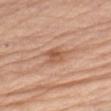anatomic site = the left upper arm
automated lesion analysis = a footprint of about 5 mm², a shape eccentricity near 0.8, and a symmetry-axis asymmetry near 0.2; a border-irregularity index near 2.5/10, a color-variation rating of about 4.5/10, and radial color variation of about 1.5
imaging modality = ~15 mm tile from a whole-body skin photo
patient = female, aged 63–67
lesion diameter = ~3.5 mm (longest diameter)